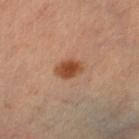follow-up = catalogued during a skin exam; not biopsied
image source = 15 mm crop, total-body photography
location = the right leg
size = about 3 mm
lighting = cross-polarized illumination
patient = female, about 40 years old